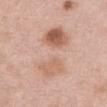The lesion was tiled from a total-body skin photograph and was not biopsied. The lesion is located on the chest. The lesion-visualizer software estimated a border-irregularity rating of about 5/10, a within-lesion color-variation index near 8.5/10, and radial color variation of about 3.5. The software also gave a classifier nevus-likeness of about 45/100 and a lesion-detection confidence of about 100/100. A 15 mm close-up tile from a total-body photography series done for melanoma screening. A female patient, roughly 50 years of age. The recorded lesion diameter is about 9 mm.